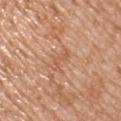follow-up = catalogued during a skin exam; not biopsied | image source = total-body-photography crop, ~15 mm field of view | location = the chest | lesion size = about 2.5 mm | lighting = white-light | patient = male, aged 48 to 52 | automated lesion analysis = a border-irregularity rating of about 7/10, internal color variation of about 0 on a 0–10 scale, and a peripheral color-asymmetry measure near 0; lesion-presence confidence of about 100/100.